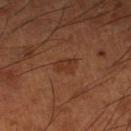{"biopsy_status": "not biopsied; imaged during a skin examination", "patient": {"sex": "male", "age_approx": 65}, "lighting": "cross-polarized", "lesion_size": {"long_diameter_mm_approx": 2.5}, "site": "left lower leg", "image": {"source": "total-body photography crop", "field_of_view_mm": 15}}